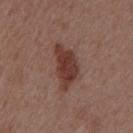<tbp_lesion>
  <image>
    <source>total-body photography crop</source>
    <field_of_view_mm>15</field_of_view_mm>
  </image>
  <lighting>white-light</lighting>
  <site>chest</site>
  <lesion_size>
    <long_diameter_mm_approx>5.5</long_diameter_mm_approx>
  </lesion_size>
  <patient>
    <sex>male</sex>
    <age_approx>55</age_approx>
  </patient>
</tbp_lesion>